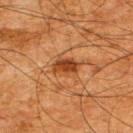Imaged during a routine full-body skin examination; the lesion was not biopsied and no histopathology is available. A roughly 15 mm field-of-view crop from a total-body skin photograph. The patient is a male in their mid-60s. The lesion's longest dimension is about 3.5 mm. An algorithmic analysis of the crop reported a mean CIELAB color near L≈36 a*≈24 b*≈35, roughly 11 lightness units darker than nearby skin, and a normalized border contrast of about 9.5. And it measured a classifier nevus-likeness of about 80/100 and a lesion-detection confidence of about 100/100. Located on the upper back.